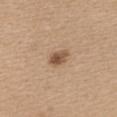| feature | finding |
|---|---|
| workup | imaged on a skin check; not biopsied |
| site | the upper back |
| diameter | ~3 mm (longest diameter) |
| patient | female, aged 58 to 62 |
| TBP lesion metrics | a lesion area of about 4.5 mm², an eccentricity of roughly 0.75, and a shape-asymmetry score of about 0.25 (0 = symmetric) |
| image source | 15 mm crop, total-body photography |
| tile lighting | white-light illumination |